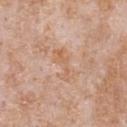biopsy status: no biopsy performed (imaged during a skin exam)
anatomic site: the chest
lesion diameter: about 4.5 mm
illumination: white-light illumination
subject: male, about 65 years old
imaging modality: ~15 mm crop, total-body skin-cancer survey